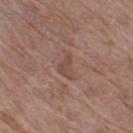Impression: The lesion was tiled from a total-body skin photograph and was not biopsied. Acquisition and patient details: The tile uses white-light illumination. A 15 mm close-up tile from a total-body photography series done for melanoma screening. A female patient aged approximately 70. The recorded lesion diameter is about 3.5 mm. Located on the right thigh.